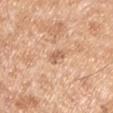Background:
A 15 mm close-up extracted from a 3D total-body photography capture. Automated tile analysis of the lesion measured a within-lesion color-variation index near 1/10 and peripheral color asymmetry of about 0.5. It also reported an automated nevus-likeness rating near 0 out of 100 and a detector confidence of about 100 out of 100 that the crop contains a lesion. The lesion is on the left upper arm. The patient is a male in their mid- to late 50s. The lesion's longest dimension is about 2.5 mm.